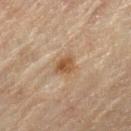biopsy status: no biopsy performed (imaged during a skin exam) | size: about 2.5 mm | patient: female, aged 78–82 | location: the right lower leg | imaging modality: 15 mm crop, total-body photography | illumination: cross-polarized.